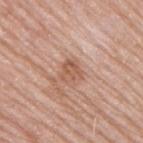{
  "site": "right upper arm",
  "image": {
    "source": "total-body photography crop",
    "field_of_view_mm": 15
  },
  "patient": {
    "sex": "male",
    "age_approx": 75
  }
}